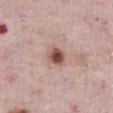Captured during whole-body skin photography for melanoma surveillance; the lesion was not biopsied.
A roughly 15 mm field-of-view crop from a total-body skin photograph.
The lesion is on the abdomen.
The total-body-photography lesion software estimated an average lesion color of about L≈51 a*≈22 b*≈24 (CIELAB), a lesion–skin lightness drop of about 16, and a normalized lesion–skin contrast near 10.5.
The recorded lesion diameter is about 2.5 mm.
A male subject roughly 75 years of age.
The tile uses white-light illumination.